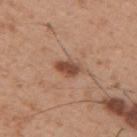Q: Is there a histopathology result?
A: no biopsy performed (imaged during a skin exam)
Q: What are the patient's age and sex?
A: male, in their 30s
Q: What is the anatomic site?
A: the right upper arm
Q: What is the imaging modality?
A: ~15 mm tile from a whole-body skin photo
Q: What did automated image analysis measure?
A: a lesion color around L≈47 a*≈22 b*≈30 in CIELAB, a lesion–skin lightness drop of about 13, and a normalized lesion–skin contrast near 9; a border-irregularity index near 3/10, a color-variation rating of about 2.5/10, and a peripheral color-asymmetry measure near 1